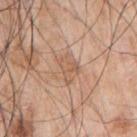Findings:
- biopsy status: total-body-photography surveillance lesion; no biopsy
- image: 15 mm crop, total-body photography
- size: ≈3 mm
- location: the left arm
- lighting: white-light
- subject: male, in their 70s